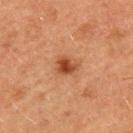workup = total-body-photography surveillance lesion; no biopsy
illumination = cross-polarized illumination
anatomic site = the upper back
acquisition = total-body-photography crop, ~15 mm field of view
patient = male, roughly 50 years of age